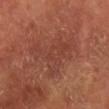About 6.5 mm across.
The tile uses cross-polarized illumination.
From the leg.
Automated tile analysis of the lesion measured a mean CIELAB color near L≈39 a*≈24 b*≈27, a lesion–skin lightness drop of about 5, and a normalized lesion–skin contrast near 4.5.
A male subject aged approximately 55.
A 15 mm close-up extracted from a 3D total-body photography capture.